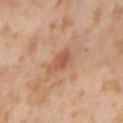follow-up=catalogued during a skin exam; not biopsied | patient=female, roughly 55 years of age | lighting=cross-polarized | imaging modality=~15 mm tile from a whole-body skin photo | anatomic site=the leg.